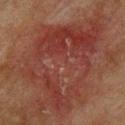Recorded during total-body skin imaging; not selected for excision or biopsy. A male patient, aged 73–77. Located on the back. A 15 mm crop from a total-body photograph taken for skin-cancer surveillance.